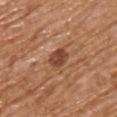Assessment: Recorded during total-body skin imaging; not selected for excision or biopsy. Acquisition and patient details: On the upper back. A 15 mm close-up extracted from a 3D total-body photography capture. An algorithmic analysis of the crop reported an average lesion color of about L≈44 a*≈23 b*≈31 (CIELAB) and roughly 12 lightness units darker than nearby skin. It also reported an automated nevus-likeness rating near 65 out of 100 and lesion-presence confidence of about 100/100. The tile uses white-light illumination. The patient is a male approximately 70 years of age. Approximately 3 mm at its widest.